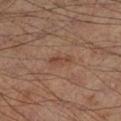Imaged during a routine full-body skin examination; the lesion was not biopsied and no histopathology is available.
This is a cross-polarized tile.
A roughly 15 mm field-of-view crop from a total-body skin photograph.
From the left lower leg.
Longest diameter approximately 3 mm.
A male patient, about 65 years old.